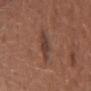The lesion was tiled from a total-body skin photograph and was not biopsied. The patient is a female roughly 60 years of age. The lesion is located on the head or neck. Automated tile analysis of the lesion measured a footprint of about 5.5 mm², an outline eccentricity of about 0.8 (0 = round, 1 = elongated), and two-axis asymmetry of about 0.3. The software also gave a border-irregularity index near 3.5/10, a color-variation rating of about 2.5/10, and radial color variation of about 0.5. And it measured a lesion-detection confidence of about 100/100. A 15 mm close-up extracted from a 3D total-body photography capture. Captured under white-light illumination.